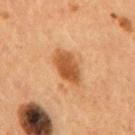The lesion was photographed on a routine skin check and not biopsied; there is no pathology result.
The lesion's longest dimension is about 4.5 mm.
The patient is a male aged 53–57.
Located on the mid back.
This is a cross-polarized tile.
A region of skin cropped from a whole-body photographic capture, roughly 15 mm wide.
The lesion-visualizer software estimated an area of roughly 8.5 mm², a shape eccentricity near 0.8, and a symmetry-axis asymmetry near 0.15. The analysis additionally found a lesion color around L≈49 a*≈24 b*≈39 in CIELAB, roughly 13 lightness units darker than nearby skin, and a normalized lesion–skin contrast near 9.5. The software also gave a border-irregularity index near 2/10, a color-variation rating of about 3.5/10, and peripheral color asymmetry of about 1. The software also gave a classifier nevus-likeness of about 95/100 and lesion-presence confidence of about 100/100.